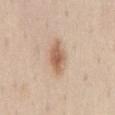The lesion was tiled from a total-body skin photograph and was not biopsied.
Automated image analysis of the tile measured a border-irregularity index near 1.5/10 and internal color variation of about 4.5 on a 0–10 scale.
Located on the lower back.
Imaged with white-light lighting.
Cropped from a total-body skin-imaging series; the visible field is about 15 mm.
A male subject aged 73–77.